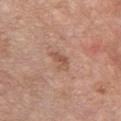{"biopsy_status": "not biopsied; imaged during a skin examination", "patient": {"sex": "male", "age_approx": 60}, "image": {"source": "total-body photography crop", "field_of_view_mm": 15}, "site": "front of the torso"}